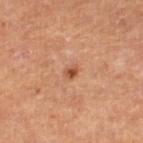Q: Was this lesion biopsied?
A: no biopsy performed (imaged during a skin exam)
Q: Where on the body is the lesion?
A: the right lower leg
Q: What lighting was used for the tile?
A: cross-polarized
Q: What are the patient's age and sex?
A: male, in their mid- to late 80s or older
Q: What kind of image is this?
A: ~15 mm crop, total-body skin-cancer survey